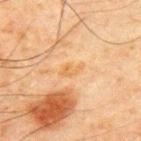follow-up: total-body-photography surveillance lesion; no biopsy
lesion diameter: ≈3 mm
body site: the chest
patient: male, in their 70s
lighting: cross-polarized
image: ~15 mm crop, total-body skin-cancer survey